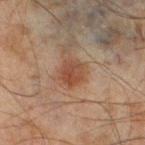| key | value |
|---|---|
| follow-up | imaged on a skin check; not biopsied |
| lighting | cross-polarized illumination |
| body site | the left lower leg |
| image source | ~15 mm crop, total-body skin-cancer survey |
| patient | male, about 45 years old |